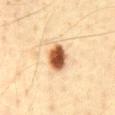No biopsy was performed on this lesion — it was imaged during a full skin examination and was not determined to be concerning. This image is a 15 mm lesion crop taken from a total-body photograph. A male patient, about 40 years old. Located on the abdomen.